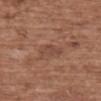- biopsy status — no biopsy performed (imaged during a skin exam)
- site — the upper back
- lighting — white-light illumination
- image-analysis metrics — an outline eccentricity of about 0.8 (0 = round, 1 = elongated) and two-axis asymmetry of about 0.4; a color-variation rating of about 1/10 and peripheral color asymmetry of about 0.5
- lesion diameter — about 2.5 mm
- image — ~15 mm crop, total-body skin-cancer survey
- patient — female, in their mid- to late 70s